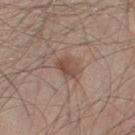The lesion is located on the right thigh. Longest diameter approximately 2.5 mm. Automated image analysis of the tile measured a mean CIELAB color near L≈48 a*≈18 b*≈26, a lesion–skin lightness drop of about 9, and a lesion-to-skin contrast of about 7 (normalized; higher = more distinct). The analysis additionally found internal color variation of about 3 on a 0–10 scale and radial color variation of about 1. The analysis additionally found an automated nevus-likeness rating near 50 out of 100 and a detector confidence of about 100 out of 100 that the crop contains a lesion. A region of skin cropped from a whole-body photographic capture, roughly 15 mm wide. The patient is a male aged approximately 30.